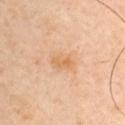Clinical impression:
Captured during whole-body skin photography for melanoma surveillance; the lesion was not biopsied.
Acquisition and patient details:
On the arm. The lesion-visualizer software estimated a border-irregularity index near 2/10 and a color-variation rating of about 3/10. It also reported an automated nevus-likeness rating near 0 out of 100. The patient is a male approximately 45 years of age. A 15 mm crop from a total-body photograph taken for skin-cancer surveillance.